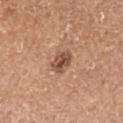follow-up = total-body-photography surveillance lesion; no biopsy
image source = ~15 mm crop, total-body skin-cancer survey
body site = the left lower leg
size = ≈2.5 mm
patient = male, aged 58–62
lighting = cross-polarized illumination